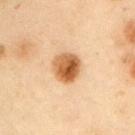biopsy_status: not biopsied; imaged during a skin examination
lesion_size:
  long_diameter_mm_approx: 3.5
image:
  source: total-body photography crop
  field_of_view_mm: 15
patient:
  sex: male
  age_approx: 55
site: left upper arm
automated_metrics:
  vs_skin_darker_L: 15.0
  vs_skin_contrast_norm: 11.0
  nevus_likeness_0_100: 100
  lesion_detection_confidence_0_100: 100